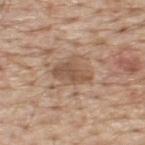No biopsy was performed on this lesion — it was imaged during a full skin examination and was not determined to be concerning. A male patient, approximately 70 years of age. A 15 mm close-up extracted from a 3D total-body photography capture. The tile uses white-light illumination. The lesion is on the upper back. The recorded lesion diameter is about 4.5 mm.